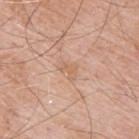This lesion was catalogued during total-body skin photography and was not selected for biopsy. From the upper back. A lesion tile, about 15 mm wide, cut from a 3D total-body photograph. Captured under white-light illumination. A male patient, aged approximately 65. About 2.5 mm across.